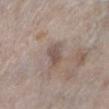notes: imaged on a skin check; not biopsied